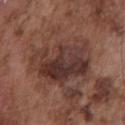image source: ~15 mm tile from a whole-body skin photo | size: ≈7.5 mm | site: the chest | subject: male, approximately 75 years of age | TBP lesion metrics: an area of roughly 29 mm² and an eccentricity of roughly 0.7; a border-irregularity index near 4.5/10, a within-lesion color-variation index near 7.5/10, and a peripheral color-asymmetry measure near 2.5; a nevus-likeness score of about 0/100 and a detector confidence of about 90 out of 100 that the crop contains a lesion.